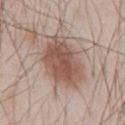  biopsy_status: not biopsied; imaged during a skin examination
  lighting: white-light
  image:
    source: total-body photography crop
    field_of_view_mm: 15
  patient:
    sex: male
    age_approx: 45
  automated_metrics:
    area_mm2_approx: 22.0
    eccentricity: 0.75
    shape_asymmetry: 0.2
    color_variation_0_10: 4.5
    peripheral_color_asymmetry: 1.5
    nevus_likeness_0_100: 100
  site: abdomen
  lesion_size:
    long_diameter_mm_approx: 6.5